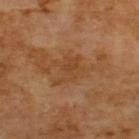biopsy status — catalogued during a skin exam; not biopsied
size — about 4 mm
subject — male, aged around 65
site — the upper back
acquisition — 15 mm crop, total-body photography